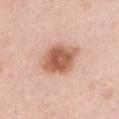workup: no biopsy performed (imaged during a skin exam) | diameter: ≈4.5 mm | imaging modality: total-body-photography crop, ~15 mm field of view | location: the chest | subject: female, aged approximately 40 | lighting: white-light.